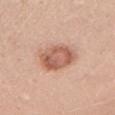A male subject about 50 years old. The lesion is on the right upper arm. A 15 mm close-up extracted from a 3D total-body photography capture.